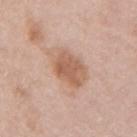Assessment:
Recorded during total-body skin imaging; not selected for excision or biopsy.
Clinical summary:
The lesion is on the right upper arm. Automated image analysis of the tile measured border irregularity of about 3 on a 0–10 scale and a within-lesion color-variation index near 4/10. A female subject aged 48 to 52. A lesion tile, about 15 mm wide, cut from a 3D total-body photograph. The lesion's longest dimension is about 5 mm. This is a white-light tile.